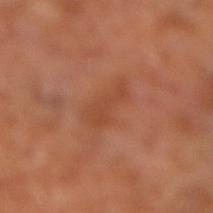| feature | finding |
|---|---|
| image | total-body-photography crop, ~15 mm field of view |
| lesion size | ~4.5 mm (longest diameter) |
| lighting | cross-polarized |
| TBP lesion metrics | a lesion area of about 7.5 mm², a shape eccentricity near 0.9, and a symmetry-axis asymmetry near 0.4; a border-irregularity index near 5/10 and a peripheral color-asymmetry measure near 0.5; a classifier nevus-likeness of about 0/100 and a lesion-detection confidence of about 100/100 |
| subject | roughly 65 years of age |
| body site | the right lower leg |